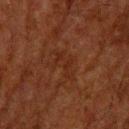This lesion was catalogued during total-body skin photography and was not selected for biopsy. The lesion is on the back. Measured at roughly 4 mm in maximum diameter. The subject is a male about 60 years old. A roughly 15 mm field-of-view crop from a total-body skin photograph.